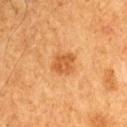Captured during whole-body skin photography for melanoma surveillance; the lesion was not biopsied. A male patient, aged around 60. About 2.5 mm across. Automated tile analysis of the lesion measured a lesion color around L≈53 a*≈27 b*≈43 in CIELAB and a normalized border contrast of about 7. It also reported border irregularity of about 2 on a 0–10 scale, internal color variation of about 2 on a 0–10 scale, and radial color variation of about 1. The tile uses cross-polarized illumination. This image is a 15 mm lesion crop taken from a total-body photograph. On the arm.